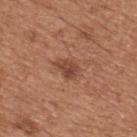{"biopsy_status": "not biopsied; imaged during a skin examination", "patient": {"sex": "male", "age_approx": 65}, "lighting": "white-light", "site": "upper back", "image": {"source": "total-body photography crop", "field_of_view_mm": 15}}